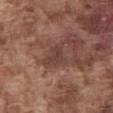Recorded during total-body skin imaging; not selected for excision or biopsy.
A male patient, in their mid-70s.
The tile uses white-light illumination.
A region of skin cropped from a whole-body photographic capture, roughly 15 mm wide.
The lesion-visualizer software estimated a mean CIELAB color near L≈40 a*≈19 b*≈22 and a normalized border contrast of about 5.5. It also reported a color-variation rating of about 2.5/10. And it measured a nevus-likeness score of about 0/100 and a detector confidence of about 65 out of 100 that the crop contains a lesion.
Measured at roughly 2.5 mm in maximum diameter.
Located on the abdomen.